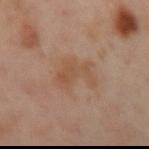Q: Is there a histopathology result?
A: total-body-photography surveillance lesion; no biopsy
Q: What kind of image is this?
A: 15 mm crop, total-body photography
Q: What is the anatomic site?
A: the left arm
Q: What is the lesion's diameter?
A: about 5 mm
Q: How was the tile lit?
A: cross-polarized illumination
Q: Patient demographics?
A: female, aged 38–42